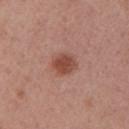Q: Was this lesion biopsied?
A: imaged on a skin check; not biopsied
Q: Automated lesion metrics?
A: a lesion area of about 6.5 mm², a shape eccentricity near 0.45, and two-axis asymmetry of about 0.15; a lesion color around L≈48 a*≈24 b*≈28 in CIELAB, a lesion–skin lightness drop of about 11, and a lesion-to-skin contrast of about 8 (normalized; higher = more distinct); a border-irregularity index near 1.5/10 and internal color variation of about 3 on a 0–10 scale
Q: How was the tile lit?
A: white-light illumination
Q: Who is the patient?
A: female, aged 48 to 52
Q: What kind of image is this?
A: 15 mm crop, total-body photography
Q: What is the anatomic site?
A: the left upper arm